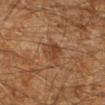Captured during whole-body skin photography for melanoma surveillance; the lesion was not biopsied.